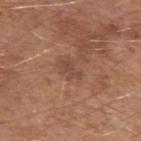Recorded during total-body skin imaging; not selected for excision or biopsy.
A region of skin cropped from a whole-body photographic capture, roughly 15 mm wide.
The lesion is on the left upper arm.
A male patient aged 63–67.
Approximately 3 mm at its widest.
An algorithmic analysis of the crop reported a footprint of about 3.5 mm², an eccentricity of roughly 0.85, and a symmetry-axis asymmetry near 0.4. And it measured a lesion-detection confidence of about 100/100.
The tile uses white-light illumination.